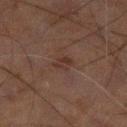The lesion was tiled from a total-body skin photograph and was not biopsied. Cropped from a whole-body photographic skin survey; the tile spans about 15 mm. The lesion's longest dimension is about 2.5 mm. This is a cross-polarized tile. From the left lower leg. A male subject roughly 60 years of age. Automated image analysis of the tile measured an area of roughly 3.5 mm², an outline eccentricity of about 0.8 (0 = round, 1 = elongated), and a shape-asymmetry score of about 0.25 (0 = symmetric). The analysis additionally found a normalized border contrast of about 5.5. The software also gave border irregularity of about 3 on a 0–10 scale, a color-variation rating of about 1.5/10, and a peripheral color-asymmetry measure near 0.5. The software also gave a lesion-detection confidence of about 100/100.